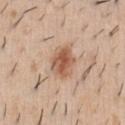{"biopsy_status": "not biopsied; imaged during a skin examination", "lighting": "white-light", "patient": {"sex": "male", "age_approx": 40}, "site": "front of the torso", "image": {"source": "total-body photography crop", "field_of_view_mm": 15}, "lesion_size": {"long_diameter_mm_approx": 4.0}, "automated_metrics": {"nevus_likeness_0_100": 90, "lesion_detection_confidence_0_100": 100}}